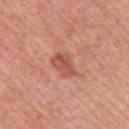| key | value |
|---|---|
| notes | no biopsy performed (imaged during a skin exam) |
| illumination | white-light |
| body site | the right upper arm |
| image source | total-body-photography crop, ~15 mm field of view |
| lesion size | about 3.5 mm |
| subject | female, in their mid- to late 50s |